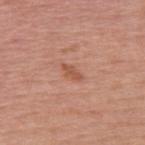Part of a total-body skin-imaging series; this lesion was reviewed on a skin check and was not flagged for biopsy. The lesion is on the upper back. A close-up tile cropped from a whole-body skin photograph, about 15 mm across. A male patient, aged 53–57.